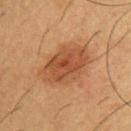From the back. Measured at roughly 6.5 mm in maximum diameter. Cropped from a whole-body photographic skin survey; the tile spans about 15 mm. This is a cross-polarized tile. A male patient, approximately 55 years of age.